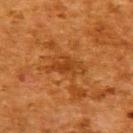biopsy_status: not biopsied; imaged during a skin examination
patient:
  sex: female
  age_approx: 50
image:
  source: total-body photography crop
  field_of_view_mm: 15
automated_metrics:
  area_mm2_approx: 8.0
  eccentricity: 0.85
  shape_asymmetry: 0.4
  border_irregularity_0_10: 4.5
  color_variation_0_10: 3.0
  peripheral_color_asymmetry: 1.0
  nevus_likeness_0_100: 0
  lesion_detection_confidence_0_100: 95
lighting: cross-polarized
site: back
lesion_size:
  long_diameter_mm_approx: 5.0